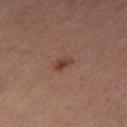Case summary:
– follow-up · total-body-photography surveillance lesion; no biopsy
– subject · female, about 60 years old
– illumination · cross-polarized
– anatomic site · the left lower leg
– image source · total-body-photography crop, ~15 mm field of view